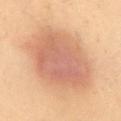Clinical impression: Part of a total-body skin-imaging series; this lesion was reviewed on a skin check and was not flagged for biopsy. Image and clinical context: Measured at roughly 8.5 mm in maximum diameter. Cropped from a total-body skin-imaging series; the visible field is about 15 mm. This is a cross-polarized tile. On the abdomen. A female subject aged approximately 40.